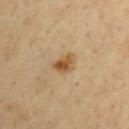| key | value |
|---|---|
| notes | catalogued during a skin exam; not biopsied |
| subject | male, in their mid-60s |
| body site | the arm |
| image source | ~15 mm tile from a whole-body skin photo |
| lighting | cross-polarized illumination |
| automated metrics | a footprint of about 5.5 mm² and a shape eccentricity near 0.65; a border-irregularity rating of about 3/10 and a color-variation rating of about 5/10; an automated nevus-likeness rating near 90 out of 100 |